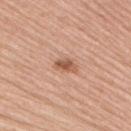| field | value |
|---|---|
| lesion diameter | ≈2.5 mm |
| anatomic site | the arm |
| illumination | white-light illumination |
| automated metrics | a mean CIELAB color near L≈56 a*≈23 b*≈32, a lesion–skin lightness drop of about 12, and a normalized lesion–skin contrast near 8; a border-irregularity index near 3/10 and a color-variation rating of about 2/10; lesion-presence confidence of about 100/100 |
| acquisition | ~15 mm tile from a whole-body skin photo |
| patient | female, approximately 60 years of age |